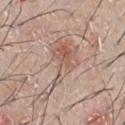{
  "image": {
    "source": "total-body photography crop",
    "field_of_view_mm": 15
  },
  "patient": {
    "sex": "male",
    "age_approx": 65
  },
  "lesion_size": {
    "long_diameter_mm_approx": 7.0
  },
  "site": "chest",
  "automated_metrics": {
    "eccentricity": 0.85,
    "shape_asymmetry": 0.65,
    "cielab_L": 57,
    "cielab_a": 18,
    "cielab_b": 26,
    "vs_skin_contrast_norm": 5.5,
    "nevus_likeness_0_100": 0,
    "lesion_detection_confidence_0_100": 100
  },
  "lighting": "white-light"
}